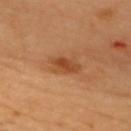Impression:
The lesion was tiled from a total-body skin photograph and was not biopsied.
Clinical summary:
Imaged with cross-polarized lighting. A female patient aged approximately 60. A 15 mm crop from a total-body photograph taken for skin-cancer surveillance. Automated image analysis of the tile measured an area of roughly 6 mm², a shape eccentricity near 0.85, and two-axis asymmetry of about 0.15. The analysis additionally found a border-irregularity rating of about 2/10 and a within-lesion color-variation index near 2.5/10. And it measured a nevus-likeness score of about 70/100 and a lesion-detection confidence of about 100/100. The lesion is located on the chest.